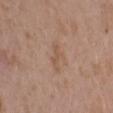Impression: The lesion was photographed on a routine skin check and not biopsied; there is no pathology result. Context: On the arm. Cropped from a total-body skin-imaging series; the visible field is about 15 mm. The total-body-photography lesion software estimated a mean CIELAB color near L≈54 a*≈18 b*≈30, a lesion–skin lightness drop of about 6, and a lesion-to-skin contrast of about 5 (normalized; higher = more distinct). It also reported a border-irregularity rating of about 5.5/10, a within-lesion color-variation index near 0/10, and a peripheral color-asymmetry measure near 0. The recorded lesion diameter is about 3 mm. A female patient, approximately 35 years of age.